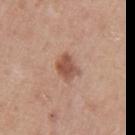Findings:
- size: ~3 mm (longest diameter)
- acquisition: total-body-photography crop, ~15 mm field of view
- site: the left upper arm
- automated metrics: an outline eccentricity of about 0.55 (0 = round, 1 = elongated) and a symmetry-axis asymmetry near 0.15
- patient: female, aged around 45